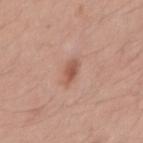workup — imaged on a skin check; not biopsied
subject — male, aged approximately 40
anatomic site — the right upper arm
image — total-body-photography crop, ~15 mm field of view
lesion size — about 2.5 mm
illumination — white-light illumination